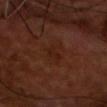Assessment:
This lesion was catalogued during total-body skin photography and was not selected for biopsy.
Acquisition and patient details:
A roughly 15 mm field-of-view crop from a total-body skin photograph. This is a cross-polarized tile. A male subject aged around 70. The lesion is on the chest. Longest diameter approximately 3 mm.